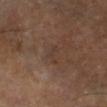  biopsy_status: not biopsied; imaged during a skin examination
  lighting: cross-polarized
  image:
    source: total-body photography crop
    field_of_view_mm: 15
  site: left lower leg
  patient:
    sex: female
    age_approx: 75
  lesion_size:
    long_diameter_mm_approx: 3.5